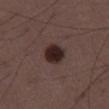illumination = white-light illumination; subject = male, aged around 50; anatomic site = the right thigh; size = ~3 mm (longest diameter); image source = 15 mm crop, total-body photography.